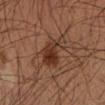| key | value |
|---|---|
| workup | catalogued during a skin exam; not biopsied |
| diameter | ≈5.5 mm |
| subject | male, approximately 35 years of age |
| imaging modality | total-body-photography crop, ~15 mm field of view |
| location | the arm |
| automated metrics | an automated nevus-likeness rating near 100 out of 100 and a detector confidence of about 100 out of 100 that the crop contains a lesion |
| lighting | cross-polarized illumination |